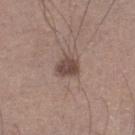Recorded during total-body skin imaging; not selected for excision or biopsy.
A male patient, in their mid-50s.
On the right lower leg.
Captured under white-light illumination.
Cropped from a whole-body photographic skin survey; the tile spans about 15 mm.
The total-body-photography lesion software estimated a nevus-likeness score of about 55/100 and a lesion-detection confidence of about 100/100.
Measured at roughly 3 mm in maximum diameter.